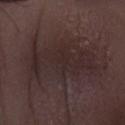  biopsy_status: not biopsied; imaged during a skin examination
  patient:
    sex: male
    age_approx: 50
  image:
    source: total-body photography crop
    field_of_view_mm: 15
  automated_metrics:
    area_mm2_approx: 32.0
    eccentricity: 0.9
    shape_asymmetry: 0.35
    border_irregularity_0_10: 7.5
    color_variation_0_10: 3.0
    peripheral_color_asymmetry: 1.0
    nevus_likeness_0_100: 0
    lesion_detection_confidence_0_100: 80